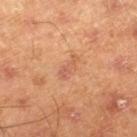* biopsy status — total-body-photography surveillance lesion; no biopsy
* tile lighting — cross-polarized
* automated metrics — a lesion area of about 4 mm² and a symmetry-axis asymmetry near 0.35; a mean CIELAB color near L≈59 a*≈25 b*≈35, a lesion–skin lightness drop of about 7, and a lesion-to-skin contrast of about 4.5 (normalized; higher = more distinct); a border-irregularity index near 3.5/10 and peripheral color asymmetry of about 0.5; an automated nevus-likeness rating near 5 out of 100 and a detector confidence of about 100 out of 100 that the crop contains a lesion
* image — total-body-photography crop, ~15 mm field of view
* site — the right lower leg
* subject — male, roughly 45 years of age
* size — about 3.5 mm